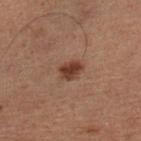Clinical impression: No biopsy was performed on this lesion — it was imaged during a full skin examination and was not determined to be concerning.